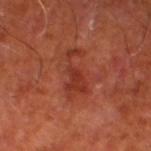workup = imaged on a skin check; not biopsied | body site = the leg | tile lighting = cross-polarized | imaging modality = total-body-photography crop, ~15 mm field of view | subject = male, approximately 65 years of age | lesion diameter = ≈5.5 mm | automated lesion analysis = a shape eccentricity near 0.9 and two-axis asymmetry of about 0.55.